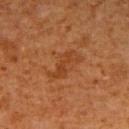anatomic site = the right upper arm
image source = total-body-photography crop, ~15 mm field of view
automated lesion analysis = a lesion–skin lightness drop of about 6 and a normalized lesion–skin contrast near 6; a border-irregularity rating of about 6.5/10 and a color-variation rating of about 2/10
illumination = cross-polarized illumination
subject = male, roughly 60 years of age
size = ≈4 mm